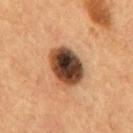<record>
  <biopsy_status>not biopsied; imaged during a skin examination</biopsy_status>
  <image>
    <source>total-body photography crop</source>
    <field_of_view_mm>15</field_of_view_mm>
  </image>
  <site>mid back</site>
  <patient>
    <sex>male</sex>
    <age_approx>55</age_approx>
  </patient>
</record>